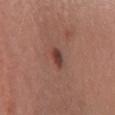No biopsy was performed on this lesion — it was imaged during a full skin examination and was not determined to be concerning.
Imaged with white-light lighting.
A lesion tile, about 15 mm wide, cut from a 3D total-body photograph.
The patient is a female about 35 years old.
About 3 mm across.
On the chest.
An algorithmic analysis of the crop reported a lesion color around L≈41 a*≈23 b*≈24 in CIELAB, about 11 CIELAB-L* units darker than the surrounding skin, and a normalized border contrast of about 9. It also reported a border-irregularity index near 2/10 and a color-variation rating of about 4.5/10. The analysis additionally found an automated nevus-likeness rating near 80 out of 100 and lesion-presence confidence of about 100/100.